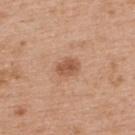<tbp_lesion>
<biopsy_status>not biopsied; imaged during a skin examination</biopsy_status>
</tbp_lesion>